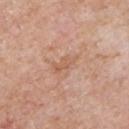  lighting: white-light
  image:
    source: total-body photography crop
    field_of_view_mm: 15
  lesion_size:
    long_diameter_mm_approx: 3.5
  patient:
    sex: male
    age_approx: 60
  site: chest